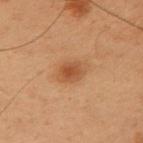workup = total-body-photography surveillance lesion; no biopsy
acquisition = total-body-photography crop, ~15 mm field of view
diameter = ~3.5 mm (longest diameter)
patient = male, aged 53–57
anatomic site = the right upper arm